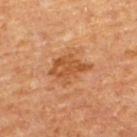Case summary:
• workup — total-body-photography surveillance lesion; no biopsy
• image — total-body-photography crop, ~15 mm field of view
• subject — male, aged around 65
• automated metrics — a footprint of about 9 mm², an outline eccentricity of about 0.7 (0 = round, 1 = elongated), and a shape-asymmetry score of about 0.35 (0 = symmetric); an average lesion color of about L≈47 a*≈24 b*≈37 (CIELAB), about 9 CIELAB-L* units darker than the surrounding skin, and a lesion-to-skin contrast of about 7.5 (normalized; higher = more distinct); peripheral color asymmetry of about 1; an automated nevus-likeness rating near 30 out of 100 and a detector confidence of about 100 out of 100 that the crop contains a lesion
• size — ~4.5 mm (longest diameter)
• anatomic site — the upper back